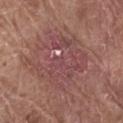follow-up=total-body-photography surveillance lesion; no biopsy.